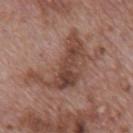• biopsy status: no biopsy performed (imaged during a skin exam)
• illumination: white-light
• image: ~15 mm tile from a whole-body skin photo
• subject: male, aged around 70
• automated metrics: an automated nevus-likeness rating near 0 out of 100 and lesion-presence confidence of about 100/100
• site: the mid back